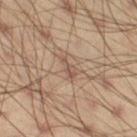Captured during whole-body skin photography for melanoma surveillance; the lesion was not biopsied.
Cropped from a whole-body photographic skin survey; the tile spans about 15 mm.
Captured under cross-polarized illumination.
About 3 mm across.
The lesion is located on the right thigh.
The patient is a male aged 38 to 42.